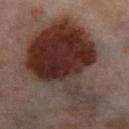{"biopsy_status": "not biopsied; imaged during a skin examination", "patient": {"sex": "female", "age_approx": 55}, "image": {"source": "total-body photography crop", "field_of_view_mm": 15}, "site": "right thigh", "lesion_size": {"long_diameter_mm_approx": 13.5}, "lighting": "cross-polarized"}